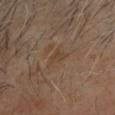Q: Is there a histopathology result?
A: total-body-photography surveillance lesion; no biopsy
Q: What is the anatomic site?
A: the head or neck
Q: Lesion size?
A: ~3 mm (longest diameter)
Q: What kind of image is this?
A: ~15 mm crop, total-body skin-cancer survey
Q: How was the tile lit?
A: cross-polarized
Q: What are the patient's age and sex?
A: male, aged 68 to 72